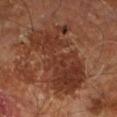The lesion was photographed on a routine skin check and not biopsied; there is no pathology result. The recorded lesion diameter is about 9 mm. A male subject aged 58–62. Automated image analysis of the tile measured an area of roughly 37 mm², an outline eccentricity of about 0.85 (0 = round, 1 = elongated), and two-axis asymmetry of about 0.4. It also reported an average lesion color of about L≈33 a*≈22 b*≈28 (CIELAB) and a normalized border contrast of about 8. The analysis additionally found a border-irregularity index near 8/10, a color-variation rating of about 4/10, and a peripheral color-asymmetry measure near 1.5. The software also gave an automated nevus-likeness rating near 0 out of 100 and a detector confidence of about 95 out of 100 that the crop contains a lesion. The lesion is on the right leg. Imaged with cross-polarized lighting. A 15 mm close-up tile from a total-body photography series done for melanoma screening.